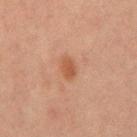  biopsy_status: not biopsied; imaged during a skin examination
  image:
    source: total-body photography crop
    field_of_view_mm: 15
  lighting: cross-polarized
  site: front of the torso
  patient:
    sex: male
    age_approx: 65
  lesion_size:
    long_diameter_mm_approx: 2.5